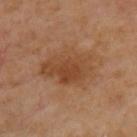{"biopsy_status": "not biopsied; imaged during a skin examination", "site": "back", "image": {"source": "total-body photography crop", "field_of_view_mm": 15}, "lighting": "cross-polarized", "automated_metrics": {"area_mm2_approx": 17.0, "eccentricity": 0.75, "shape_asymmetry": 0.2, "nevus_likeness_0_100": 0}, "lesion_size": {"long_diameter_mm_approx": 6.0}, "patient": {"sex": "male", "age_approx": 70}}